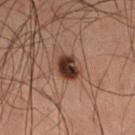biopsy status: catalogued during a skin exam; not biopsied | lighting: cross-polarized | image source: ~15 mm crop, total-body skin-cancer survey | subject: male, aged 58 to 62 | location: the leg | image-analysis metrics: peripheral color asymmetry of about 1; an automated nevus-likeness rating near 90 out of 100 and a detector confidence of about 100 out of 100 that the crop contains a lesion | lesion size: about 3 mm.